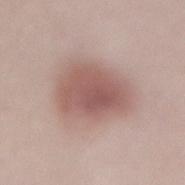This lesion was catalogued during total-body skin photography and was not selected for biopsy. The total-body-photography lesion software estimated an area of roughly 23 mm², an outline eccentricity of about 0.7 (0 = round, 1 = elongated), and two-axis asymmetry of about 0.2. This image is a 15 mm lesion crop taken from a total-body photograph. The lesion is on the lower back. The tile uses white-light illumination. The recorded lesion diameter is about 6 mm. A female subject aged around 40.